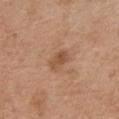follow-up: imaged on a skin check; not biopsied
size: ~2.5 mm (longest diameter)
patient: male, aged 53 to 57
imaging modality: total-body-photography crop, ~15 mm field of view
anatomic site: the front of the torso
lighting: white-light illumination